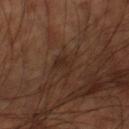Impression:
Imaged during a routine full-body skin examination; the lesion was not biopsied and no histopathology is available.